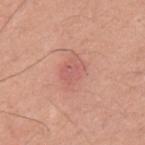Background: The recorded lesion diameter is about 4 mm. A male patient, in their mid-20s. The tile uses white-light illumination. A 15 mm close-up extracted from a 3D total-body photography capture. Automated tile analysis of the lesion measured a footprint of about 10 mm² and an outline eccentricity of about 0.7 (0 = round, 1 = elongated). The analysis additionally found border irregularity of about 2.5 on a 0–10 scale and a within-lesion color-variation index near 2.5/10. The software also gave an automated nevus-likeness rating near 5 out of 100 and lesion-presence confidence of about 100/100. Located on the upper back.